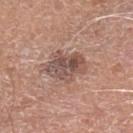Image and clinical context:
The lesion is on the left lower leg. Longest diameter approximately 5 mm. This is a white-light tile. A roughly 15 mm field-of-view crop from a total-body skin photograph. A male subject, in their 80s.